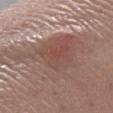| field | value |
|---|---|
| notes | no biopsy performed (imaged during a skin exam) |